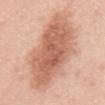workup — total-body-photography surveillance lesion; no biopsy
acquisition — total-body-photography crop, ~15 mm field of view
lesion size — about 10.5 mm
location — the abdomen
subject — male, roughly 45 years of age